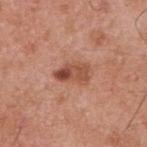Notes:
– biopsy status · imaged on a skin check; not biopsied
– location · the back
– size · ≈4 mm
– automated metrics · a mean CIELAB color near L≈50 a*≈25 b*≈31, about 12 CIELAB-L* units darker than the surrounding skin, and a normalized lesion–skin contrast near 8.5
– acquisition · ~15 mm tile from a whole-body skin photo
– subject · male, in their mid- to late 50s